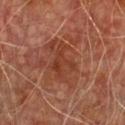biopsy_status: not biopsied; imaged during a skin examination
patient:
  sex: male
  age_approx: 75
lighting: cross-polarized
lesion_size:
  long_diameter_mm_approx: 4.5
image:
  source: total-body photography crop
  field_of_view_mm: 15
site: chest